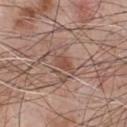follow-up: total-body-photography surveillance lesion; no biopsy | body site: the chest | subject: male, approximately 55 years of age | illumination: white-light illumination | imaging modality: total-body-photography crop, ~15 mm field of view | lesion size: about 2.5 mm.